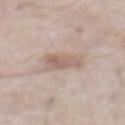* biopsy status: imaged on a skin check; not biopsied
* patient: male, roughly 75 years of age
* automated lesion analysis: an area of roughly 8 mm² and a shape eccentricity near 0.7; a lesion–skin lightness drop of about 9; an automated nevus-likeness rating near 40 out of 100 and a detector confidence of about 100 out of 100 that the crop contains a lesion
* lesion diameter: about 3.5 mm
* imaging modality: ~15 mm tile from a whole-body skin photo
* lighting: white-light illumination
* location: the abdomen